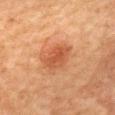Case summary:
* follow-up · total-body-photography surveillance lesion; no biopsy
* subject · female, aged around 60
* image source · ~15 mm tile from a whole-body skin photo
* illumination · cross-polarized
* size · ≈4 mm
* anatomic site · the mid back
* TBP lesion metrics · a symmetry-axis asymmetry near 0.2; about 9 CIELAB-L* units darker than the surrounding skin and a normalized border contrast of about 7; a nevus-likeness score of about 100/100